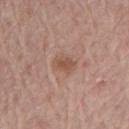Part of a total-body skin-imaging series; this lesion was reviewed on a skin check and was not flagged for biopsy. About 3 mm across. A female subject aged 68 to 72. A 15 mm close-up tile from a total-body photography series done for melanoma screening. Automated image analysis of the tile measured an outline eccentricity of about 0.75 (0 = round, 1 = elongated) and two-axis asymmetry of about 0.2. The analysis additionally found an average lesion color of about L≈52 a*≈21 b*≈29 (CIELAB), a lesion–skin lightness drop of about 8, and a normalized lesion–skin contrast near 6.5. It also reported a color-variation rating of about 2.5/10 and a peripheral color-asymmetry measure near 1. The analysis additionally found a detector confidence of about 100 out of 100 that the crop contains a lesion. Located on the left upper arm. The tile uses white-light illumination.